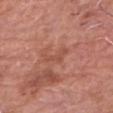{"lesion_size": {"long_diameter_mm_approx": 3.5}, "patient": {"sex": "male", "age_approx": 80}, "site": "chest", "image": {"source": "total-body photography crop", "field_of_view_mm": 15}}